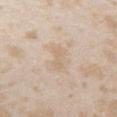Clinical impression:
Captured during whole-body skin photography for melanoma surveillance; the lesion was not biopsied.
Image and clinical context:
The subject is a female approximately 25 years of age. This is a white-light tile. A 15 mm close-up tile from a total-body photography series done for melanoma screening. The lesion is on the chest. The lesion's longest dimension is about 3.5 mm.